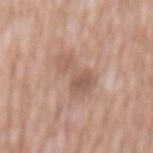follow-up = catalogued during a skin exam; not biopsied | patient = male, in their 60s | lesion diameter = about 6 mm | location = the abdomen | image source = ~15 mm tile from a whole-body skin photo.